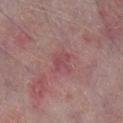<tbp_lesion>
<biopsy_status>not biopsied; imaged during a skin examination</biopsy_status>
<automated_metrics>
  <area_mm2_approx>3.0</area_mm2_approx>
  <border_irregularity_0_10>3.5</border_irregularity_0_10>
  <peripheral_color_asymmetry>0.5</peripheral_color_asymmetry>
  <nevus_likeness_0_100>0</nevus_likeness_0_100>
  <lesion_detection_confidence_0_100>100</lesion_detection_confidence_0_100>
</automated_metrics>
<image>
  <source>total-body photography crop</source>
  <field_of_view_mm>15</field_of_view_mm>
</image>
<patient>
  <sex>male</sex>
  <age_approx>75</age_approx>
</patient>
<site>right lower leg</site>
<lighting>white-light</lighting>
<lesion_size>
  <long_diameter_mm_approx>2.5</long_diameter_mm_approx>
</lesion_size>
</tbp_lesion>